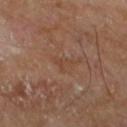Imaged during a routine full-body skin examination; the lesion was not biopsied and no histopathology is available. Captured under cross-polarized illumination. A male patient approximately 65 years of age. An algorithmic analysis of the crop reported a border-irregularity index near 6.5/10 and a within-lesion color-variation index near 0/10. And it measured a nevus-likeness score of about 0/100 and a detector confidence of about 95 out of 100 that the crop contains a lesion. The lesion is located on the left lower leg. Cropped from a whole-body photographic skin survey; the tile spans about 15 mm.